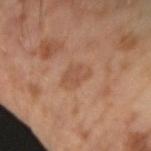Findings:
• follow-up: imaged on a skin check; not biopsied
• lesion diameter: ~3.5 mm (longest diameter)
• image source: ~15 mm crop, total-body skin-cancer survey
• illumination: cross-polarized illumination
• patient: male, aged around 65